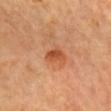  biopsy_status: not biopsied; imaged during a skin examination
  image:
    source: total-body photography crop
    field_of_view_mm: 15
  lighting: cross-polarized
  site: head or neck
  lesion_size:
    long_diameter_mm_approx: 3.0
  patient:
    sex: female
    age_approx: 65